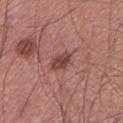The lesion was photographed on a routine skin check and not biopsied; there is no pathology result.
The lesion is located on the left lower leg.
The total-body-photography lesion software estimated a footprint of about 4.5 mm², an eccentricity of roughly 0.8, and a shape-asymmetry score of about 0.25 (0 = symmetric). It also reported a lesion–skin lightness drop of about 11 and a lesion-to-skin contrast of about 8.5 (normalized; higher = more distinct). It also reported a border-irregularity index near 2.5/10, internal color variation of about 2.5 on a 0–10 scale, and peripheral color asymmetry of about 1.
A 15 mm crop from a total-body photograph taken for skin-cancer surveillance.
The lesion's longest dimension is about 3 mm.
A male subject, aged approximately 60.
Imaged with white-light lighting.